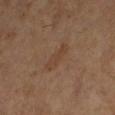Clinical impression: Captured during whole-body skin photography for melanoma surveillance; the lesion was not biopsied. Image and clinical context: Located on the left lower leg. Measured at roughly 4 mm in maximum diameter. A 15 mm close-up extracted from a 3D total-body photography capture. Automated image analysis of the tile measured a footprint of about 5 mm² and an outline eccentricity of about 0.95 (0 = round, 1 = elongated). The analysis additionally found a mean CIELAB color near L≈40 a*≈17 b*≈28, about 5 CIELAB-L* units darker than the surrounding skin, and a normalized lesion–skin contrast near 5. The software also gave border irregularity of about 3 on a 0–10 scale and a color-variation rating of about 1.5/10. The software also gave a nevus-likeness score of about 0/100 and a lesion-detection confidence of about 100/100. A female patient, approximately 65 years of age. This is a cross-polarized tile.